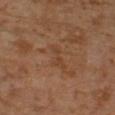Captured during whole-body skin photography for melanoma surveillance; the lesion was not biopsied. The lesion is on the left leg. A lesion tile, about 15 mm wide, cut from a 3D total-body photograph. Imaged with cross-polarized lighting. The patient is a male approximately 30 years of age.